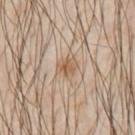{
  "biopsy_status": "not biopsied; imaged during a skin examination",
  "lighting": "cross-polarized",
  "site": "left upper arm",
  "lesion_size": {
    "long_diameter_mm_approx": 2.5
  },
  "patient": {
    "sex": "male",
    "age_approx": 40
  },
  "image": {
    "source": "total-body photography crop",
    "field_of_view_mm": 15
  },
  "automated_metrics": {
    "area_mm2_approx": 4.0,
    "eccentricity": 0.65,
    "shape_asymmetry": 0.2,
    "cielab_L": 58,
    "cielab_a": 16,
    "cielab_b": 32,
    "vs_skin_contrast_norm": 7.5,
    "border_irregularity_0_10": 2.0,
    "peripheral_color_asymmetry": 2.0
  }
}